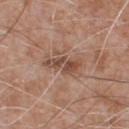| feature | finding |
|---|---|
| follow-up | imaged on a skin check; not biopsied |
| lesion size | ≈4.5 mm |
| lighting | white-light |
| image | ~15 mm tile from a whole-body skin photo |
| body site | the chest |
| subject | male, in their mid-60s |